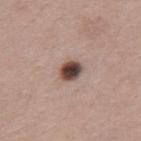biopsy status: imaged on a skin check; not biopsied | body site: the upper back | size: ~3 mm (longest diameter) | image source: total-body-photography crop, ~15 mm field of view | patient: male, in their mid-20s.